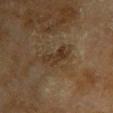| field | value |
|---|---|
| notes | catalogued during a skin exam; not biopsied |
| imaging modality | total-body-photography crop, ~15 mm field of view |
| diameter | ≈3.5 mm |
| location | the chest |
| patient | female, roughly 80 years of age |
| image-analysis metrics | a classifier nevus-likeness of about 0/100 and lesion-presence confidence of about 90/100 |
| lighting | cross-polarized illumination |